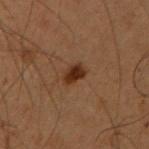The lesion was photographed on a routine skin check and not biopsied; there is no pathology result.
The lesion is on the right upper arm.
About 2.5 mm across.
A male patient, aged around 55.
Automated tile analysis of the lesion measured a lesion area of about 4 mm², an outline eccentricity of about 0.75 (0 = round, 1 = elongated), and a symmetry-axis asymmetry near 0.2. It also reported a nevus-likeness score of about 95/100 and a detector confidence of about 100 out of 100 that the crop contains a lesion.
The tile uses cross-polarized illumination.
Cropped from a total-body skin-imaging series; the visible field is about 15 mm.